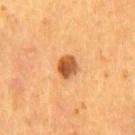Impression:
Imaged during a routine full-body skin examination; the lesion was not biopsied and no histopathology is available.
Background:
Located on the chest. A male subject aged 53–57. A lesion tile, about 15 mm wide, cut from a 3D total-body photograph.